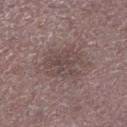This lesion was catalogued during total-body skin photography and was not selected for biopsy. The lesion is located on the left lower leg. The lesion's longest dimension is about 5 mm. A 15 mm close-up tile from a total-body photography series done for melanoma screening. A male patient approximately 50 years of age. Captured under white-light illumination.